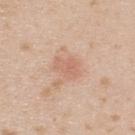Impression: Part of a total-body skin-imaging series; this lesion was reviewed on a skin check and was not flagged for biopsy. Image and clinical context: A close-up tile cropped from a whole-body skin photograph, about 15 mm across. Automated tile analysis of the lesion measured a border-irregularity index near 3/10, internal color variation of about 2 on a 0–10 scale, and peripheral color asymmetry of about 0.5. The software also gave an automated nevus-likeness rating near 5 out of 100 and a detector confidence of about 100 out of 100 that the crop contains a lesion. The lesion is located on the upper back. Approximately 3.5 mm at its widest. A male patient, in their mid-20s.